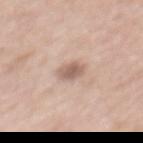{
  "biopsy_status": "not biopsied; imaged during a skin examination",
  "automated_metrics": {
    "area_mm2_approx": 4.0,
    "eccentricity": 0.6,
    "shape_asymmetry": 0.2,
    "cielab_L": 60,
    "cielab_a": 18,
    "cielab_b": 26,
    "vs_skin_darker_L": 12.0,
    "vs_skin_contrast_norm": 7.5,
    "border_irregularity_0_10": 2.0,
    "nevus_likeness_0_100": 40
  },
  "site": "mid back",
  "image": {
    "source": "total-body photography crop",
    "field_of_view_mm": 15
  },
  "patient": {
    "sex": "female",
    "age_approx": 40
  },
  "lighting": "white-light"
}